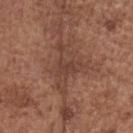Part of a total-body skin-imaging series; this lesion was reviewed on a skin check and was not flagged for biopsy. Longest diameter approximately 6 mm. A region of skin cropped from a whole-body photographic capture, roughly 15 mm wide. A male patient aged 73–77. The lesion is on the head or neck. Automated image analysis of the tile measured a footprint of about 12 mm² and an outline eccentricity of about 0.7 (0 = round, 1 = elongated). It also reported a mean CIELAB color near L≈42 a*≈20 b*≈26, a lesion–skin lightness drop of about 7, and a lesion-to-skin contrast of about 6 (normalized; higher = more distinct). And it measured internal color variation of about 3 on a 0–10 scale and peripheral color asymmetry of about 1. It also reported a classifier nevus-likeness of about 0/100 and a lesion-detection confidence of about 60/100. This is a white-light tile.